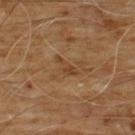{"biopsy_status": "not biopsied; imaged during a skin examination", "patient": {"sex": "male", "age_approx": 60}, "site": "chest", "image": {"source": "total-body photography crop", "field_of_view_mm": 15}, "lesion_size": {"long_diameter_mm_approx": 3.0}}